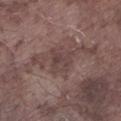biopsy_status: not biopsied; imaged during a skin examination
site: left lower leg
patient:
  sex: male
  age_approx: 75
image:
  source: total-body photography crop
  field_of_view_mm: 15
lighting: white-light